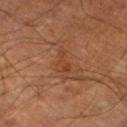Captured during whole-body skin photography for melanoma surveillance; the lesion was not biopsied. Imaged with cross-polarized lighting. From the right lower leg. An algorithmic analysis of the crop reported a lesion area of about 3.5 mm² and two-axis asymmetry of about 0.6. It also reported a lesion color around L≈38 a*≈23 b*≈31 in CIELAB and about 5 CIELAB-L* units darker than the surrounding skin. It also reported a border-irregularity rating of about 6.5/10 and radial color variation of about 0.5. It also reported a classifier nevus-likeness of about 0/100 and a detector confidence of about 100 out of 100 that the crop contains a lesion. A 15 mm close-up extracted from a 3D total-body photography capture. The subject is a male roughly 65 years of age. The lesion's longest dimension is about 3 mm.